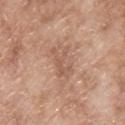| feature | finding |
|---|---|
| notes | total-body-photography surveillance lesion; no biopsy |
| lesion diameter | about 3.5 mm |
| subject | male, in their mid- to late 60s |
| illumination | white-light illumination |
| site | the upper back |
| image | 15 mm crop, total-body photography |
| image-analysis metrics | a footprint of about 5.5 mm² and two-axis asymmetry of about 0.45; a mean CIELAB color near L≈57 a*≈21 b*≈29 and roughly 7 lightness units darker than nearby skin; a lesion-detection confidence of about 100/100 |